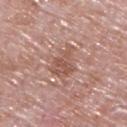Clinical impression:
The lesion was tiled from a total-body skin photograph and was not biopsied.
Acquisition and patient details:
This is a white-light tile. An algorithmic analysis of the crop reported an average lesion color of about L≈54 a*≈22 b*≈26 (CIELAB), roughly 9 lightness units darker than nearby skin, and a normalized border contrast of about 6. The software also gave a nevus-likeness score of about 0/100 and a lesion-detection confidence of about 95/100. A male patient, approximately 65 years of age. A close-up tile cropped from a whole-body skin photograph, about 15 mm across. Approximately 4 mm at its widest. On the upper back.